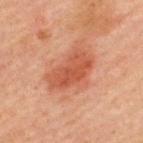Background:
The lesion's longest dimension is about 5.5 mm. A male subject, aged 58–62. The tile uses cross-polarized illumination. Automated image analysis of the tile measured a lesion area of about 14 mm², a shape eccentricity near 0.85, and a shape-asymmetry score of about 0.25 (0 = symmetric). The lesion is on the upper back. This image is a 15 mm lesion crop taken from a total-body photograph.